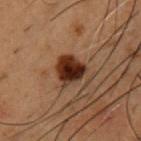Captured during whole-body skin photography for melanoma surveillance; the lesion was not biopsied.
The patient is a male approximately 50 years of age.
Automated tile analysis of the lesion measured a mean CIELAB color near L≈23 a*≈18 b*≈23, about 15 CIELAB-L* units darker than the surrounding skin, and a lesion-to-skin contrast of about 15.5 (normalized; higher = more distinct). And it measured a border-irregularity index near 2/10, a color-variation rating of about 6/10, and radial color variation of about 1.5. And it measured a classifier nevus-likeness of about 95/100 and lesion-presence confidence of about 100/100.
The tile uses cross-polarized illumination.
A 15 mm close-up tile from a total-body photography series done for melanoma screening.
Measured at roughly 3.5 mm in maximum diameter.
From the chest.